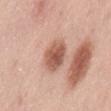This lesion was catalogued during total-body skin photography and was not selected for biopsy. Located on the back. A 15 mm crop from a total-body photograph taken for skin-cancer surveillance. This is a white-light tile. The subject is a male aged 48–52.